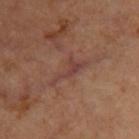Imaged during a routine full-body skin examination; the lesion was not biopsied and no histopathology is available. A 15 mm crop from a total-body photograph taken for skin-cancer surveillance. A female patient, roughly 45 years of age. Automated tile analysis of the lesion measured an eccentricity of roughly 0.9 and two-axis asymmetry of about 0.6. The software also gave a mean CIELAB color near L≈41 a*≈21 b*≈23 and roughly 6 lightness units darker than nearby skin. The analysis additionally found a border-irregularity rating of about 7.5/10, internal color variation of about 2.5 on a 0–10 scale, and a peripheral color-asymmetry measure near 1. Measured at roughly 5.5 mm in maximum diameter. The tile uses cross-polarized illumination. On the upper back.